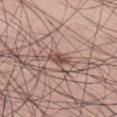Impression:
Part of a total-body skin-imaging series; this lesion was reviewed on a skin check and was not flagged for biopsy.
Acquisition and patient details:
Approximately 3 mm at its widest. A male patient, about 25 years old. A lesion tile, about 15 mm wide, cut from a 3D total-body photograph. The total-body-photography lesion software estimated a footprint of about 3.5 mm² and a shape eccentricity near 0.8. The lesion is located on the left thigh.